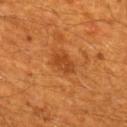Q: Is there a histopathology result?
A: total-body-photography surveillance lesion; no biopsy
Q: What lighting was used for the tile?
A: cross-polarized
Q: Patient demographics?
A: male, about 60 years old
Q: Where on the body is the lesion?
A: the mid back
Q: What is the imaging modality?
A: total-body-photography crop, ~15 mm field of view